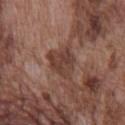Assessment: The lesion was photographed on a routine skin check and not biopsied; there is no pathology result.